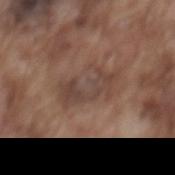Captured during whole-body skin photography for melanoma surveillance; the lesion was not biopsied. A 15 mm close-up tile from a total-body photography series done for melanoma screening. A male patient, aged 73–77. Located on the mid back.